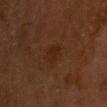Case summary:
• notes — imaged on a skin check; not biopsied
• size — ≈2.5 mm
• acquisition — total-body-photography crop, ~15 mm field of view
• subject — male, aged around 60
• location — the head or neck